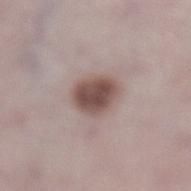The lesion was photographed on a routine skin check and not biopsied; there is no pathology result.
The subject is a female in their 50s.
Imaged with white-light lighting.
The lesion is on the left lower leg.
A 15 mm close-up tile from a total-body photography series done for melanoma screening.